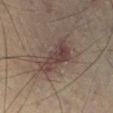Imaged during a routine full-body skin examination; the lesion was not biopsied and no histopathology is available. On the right lower leg. A region of skin cropped from a whole-body photographic capture, roughly 15 mm wide. The patient is a male about 65 years old. An algorithmic analysis of the crop reported a lesion color around L≈42 a*≈15 b*≈19 in CIELAB, about 9 CIELAB-L* units darker than the surrounding skin, and a lesion-to-skin contrast of about 8 (normalized; higher = more distinct). The analysis additionally found a classifier nevus-likeness of about 25/100 and a lesion-detection confidence of about 100/100. The recorded lesion diameter is about 6 mm. Captured under cross-polarized illumination.